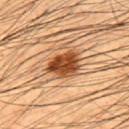follow-up=imaged on a skin check; not biopsied | lighting=cross-polarized | location=the upper back | size=about 4.5 mm | imaging modality=~15 mm crop, total-body skin-cancer survey | patient=male, in their 50s.